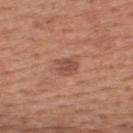| key | value |
|---|---|
| follow-up | catalogued during a skin exam; not biopsied |
| illumination | white-light illumination |
| acquisition | ~15 mm crop, total-body skin-cancer survey |
| subject | male, aged approximately 55 |
| site | the upper back |